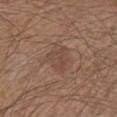Q: Was a biopsy performed?
A: imaged on a skin check; not biopsied
Q: Where on the body is the lesion?
A: the chest
Q: What is the imaging modality?
A: total-body-photography crop, ~15 mm field of view
Q: Illumination type?
A: white-light illumination
Q: What is the lesion's diameter?
A: about 3.5 mm
Q: What are the patient's age and sex?
A: male, aged around 65